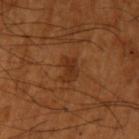Part of a total-body skin-imaging series; this lesion was reviewed on a skin check and was not flagged for biopsy. Captured under cross-polarized illumination. On the arm. Automated tile analysis of the lesion measured a lesion area of about 4.5 mm², a shape eccentricity near 0.7, and two-axis asymmetry of about 0.3. The software also gave a border-irregularity index near 3/10 and a peripheral color-asymmetry measure near 0.5. The analysis additionally found a nevus-likeness score of about 10/100 and lesion-presence confidence of about 100/100. The lesion's longest dimension is about 3 mm. A 15 mm close-up extracted from a 3D total-body photography capture. A male subject, in their mid- to late 60s.